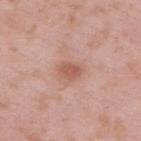No biopsy was performed on this lesion — it was imaged during a full skin examination and was not determined to be concerning. A 15 mm close-up tile from a total-body photography series done for melanoma screening. Captured under white-light illumination. The lesion is on the upper back. The patient is a male roughly 55 years of age.